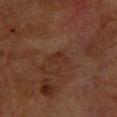| key | value |
|---|---|
| follow-up | catalogued during a skin exam; not biopsied |
| size | about 3 mm |
| lighting | cross-polarized illumination |
| site | the back |
| imaging modality | total-body-photography crop, ~15 mm field of view |
| patient | female, aged 78 to 82 |
| TBP lesion metrics | a border-irregularity index near 4/10, a color-variation rating of about 1/10, and radial color variation of about 0.5; a classifier nevus-likeness of about 0/100 and a detector confidence of about 100 out of 100 that the crop contains a lesion |